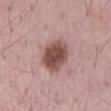| field | value |
|---|---|
| workup | catalogued during a skin exam; not biopsied |
| TBP lesion metrics | a shape eccentricity near 0.85 and two-axis asymmetry of about 0.2 |
| patient | male, about 70 years old |
| lighting | white-light |
| acquisition | total-body-photography crop, ~15 mm field of view |
| body site | the mid back |
| size | about 5.5 mm |